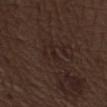The lesion was tiled from a total-body skin photograph and was not biopsied.
A close-up tile cropped from a whole-body skin photograph, about 15 mm across.
Located on the right lower leg.
The total-body-photography lesion software estimated a lesion area of about 2 mm². And it measured lesion-presence confidence of about 70/100.
Longest diameter approximately 2.5 mm.
The tile uses white-light illumination.
The subject is a male aged around 70.